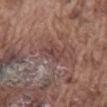| field | value |
|---|---|
| follow-up | imaged on a skin check; not biopsied |
| illumination | white-light |
| lesion size | ≈3 mm |
| image | ~15 mm crop, total-body skin-cancer survey |
| subject | male, about 75 years old |
| body site | the back |